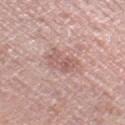Case summary:
– follow-up: no biopsy performed (imaged during a skin exam)
– patient: female, in their 40s
– image: 15 mm crop, total-body photography
– site: the right thigh